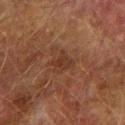The lesion was photographed on a routine skin check and not biopsied; there is no pathology result. Imaged with cross-polarized lighting. About 2.5 mm across. A 15 mm crop from a total-body photograph taken for skin-cancer surveillance. From the right upper arm. A male patient, aged 73–77.